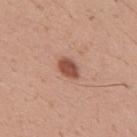Findings:
• follow-up: catalogued during a skin exam; not biopsied
• subject: male, roughly 30 years of age
• tile lighting: white-light
• acquisition: 15 mm crop, total-body photography
• location: the upper back
• image-analysis metrics: an area of roughly 4.5 mm², an outline eccentricity of about 0.75 (0 = round, 1 = elongated), and a shape-asymmetry score of about 0.15 (0 = symmetric); a border-irregularity index near 1.5/10